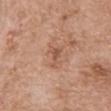workup: imaged on a skin check; not biopsied
automated lesion analysis: a footprint of about 3.5 mm², a shape eccentricity near 0.75, and two-axis asymmetry of about 0.5
illumination: white-light illumination
acquisition: total-body-photography crop, ~15 mm field of view
anatomic site: the chest
subject: male, roughly 65 years of age
lesion size: about 2.5 mm